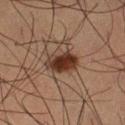workup: no biopsy performed (imaged during a skin exam) | body site: the left thigh | patient: male, about 50 years old | size: about 4 mm | image source: ~15 mm crop, total-body skin-cancer survey | tile lighting: cross-polarized illumination.